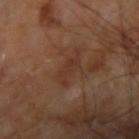Q: Is there a histopathology result?
A: imaged on a skin check; not biopsied